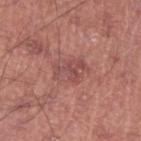The lesion was photographed on a routine skin check and not biopsied; there is no pathology result. A male patient in their mid- to late 40s. An algorithmic analysis of the crop reported an eccentricity of roughly 0.8. The analysis additionally found a border-irregularity index near 2.5/10, a within-lesion color-variation index near 4.5/10, and radial color variation of about 1.5. It also reported a nevus-likeness score of about 0/100 and lesion-presence confidence of about 100/100. On the leg. The tile uses white-light illumination. A 15 mm crop from a total-body photograph taken for skin-cancer surveillance. Measured at roughly 4 mm in maximum diameter.